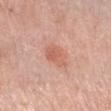Clinical impression:
Recorded during total-body skin imaging; not selected for excision or biopsy.
Background:
This is a white-light tile. A female patient approximately 65 years of age. On the arm. Automated image analysis of the tile measured a lesion area of about 6 mm² and a shape-asymmetry score of about 0.25 (0 = symmetric). The software also gave a mean CIELAB color near L≈61 a*≈26 b*≈31, about 8 CIELAB-L* units darker than the surrounding skin, and a lesion-to-skin contrast of about 6 (normalized; higher = more distinct). It also reported a classifier nevus-likeness of about 50/100 and a detector confidence of about 100 out of 100 that the crop contains a lesion. A region of skin cropped from a whole-body photographic capture, roughly 15 mm wide.